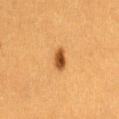Notes:
- biopsy status — total-body-photography surveillance lesion; no biopsy
- patient — female, in their 40s
- imaging modality — total-body-photography crop, ~15 mm field of view
- lighting — cross-polarized
- site — the lower back
- lesion size — ≈2.5 mm
- automated lesion analysis — a lesion color around L≈42 a*≈22 b*≈38 in CIELAB, a lesion–skin lightness drop of about 14, and a normalized lesion–skin contrast near 11; a classifier nevus-likeness of about 100/100